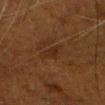Findings:
• biopsy status · imaged on a skin check; not biopsied
• acquisition · total-body-photography crop, ~15 mm field of view
• subject · male, about 75 years old
• lesion diameter · ≈2.5 mm
• illumination · cross-polarized illumination
• anatomic site · the head or neck